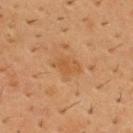Impression:
No biopsy was performed on this lesion — it was imaged during a full skin examination and was not determined to be concerning.
Image and clinical context:
Approximately 3.5 mm at its widest. A male subject aged 53 to 57. Located on the chest. Imaged with cross-polarized lighting. Cropped from a whole-body photographic skin survey; the tile spans about 15 mm.